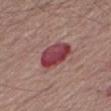Imaged during a routine full-body skin examination; the lesion was not biopsied and no histopathology is available. The total-body-photography lesion software estimated a footprint of about 11 mm², a shape eccentricity near 0.8, and two-axis asymmetry of about 0.2. The lesion is on the right thigh. A 15 mm close-up tile from a total-body photography series done for melanoma screening. Measured at roughly 4.5 mm in maximum diameter. A male patient, roughly 65 years of age.